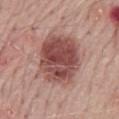| feature | finding |
|---|---|
| notes | catalogued during a skin exam; not biopsied |
| subject | male, about 70 years old |
| body site | the back |
| acquisition | total-body-photography crop, ~15 mm field of view |
| tile lighting | white-light |
| lesion diameter | about 6 mm |